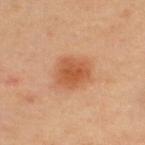Part of a total-body skin-imaging series; this lesion was reviewed on a skin check and was not flagged for biopsy. A 15 mm close-up extracted from a 3D total-body photography capture. A male subject, aged 43–47. Longest diameter approximately 3.5 mm. Automated tile analysis of the lesion measured an eccentricity of roughly 0.5 and a symmetry-axis asymmetry near 0.2. It also reported a border-irregularity index near 2/10 and internal color variation of about 3 on a 0–10 scale. The lesion is on the upper back. The tile uses cross-polarized illumination.